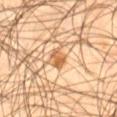Recorded during total-body skin imaging; not selected for excision or biopsy.